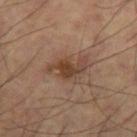This lesion was catalogued during total-body skin photography and was not selected for biopsy.
The subject is a male aged around 60.
The lesion is located on the right thigh.
The lesion-visualizer software estimated a shape eccentricity near 0.85 and a symmetry-axis asymmetry near 0.4.
A 15 mm close-up tile from a total-body photography series done for melanoma screening.